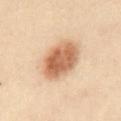Assessment:
Captured during whole-body skin photography for melanoma surveillance; the lesion was not biopsied.
Background:
On the front of the torso. The subject is a female aged 28–32. A region of skin cropped from a whole-body photographic capture, roughly 15 mm wide. Longest diameter approximately 5 mm.